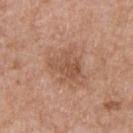No biopsy was performed on this lesion — it was imaged during a full skin examination and was not determined to be concerning.
The patient is a male roughly 50 years of age.
Cropped from a total-body skin-imaging series; the visible field is about 15 mm.
The lesion is located on the chest.